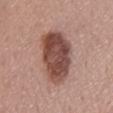– notes · catalogued during a skin exam; not biopsied
– patient · male, aged approximately 55
– diameter · about 8 mm
– anatomic site · the mid back
– acquisition · ~15 mm tile from a whole-body skin photo
– illumination · white-light illumination